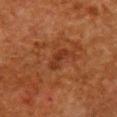Assessment: Recorded during total-body skin imaging; not selected for excision or biopsy. Acquisition and patient details: A female patient aged approximately 50. A roughly 15 mm field-of-view crop from a total-body skin photograph. Longest diameter approximately 3.5 mm. The tile uses cross-polarized illumination. An algorithmic analysis of the crop reported border irregularity of about 4 on a 0–10 scale, internal color variation of about 2 on a 0–10 scale, and peripheral color asymmetry of about 0.5. The lesion is located on the front of the torso.